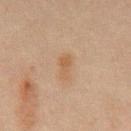Case summary:
– workup: imaged on a skin check; not biopsied
– patient: male, about 45 years old
– illumination: cross-polarized illumination
– diameter: about 3 mm
– imaging modality: total-body-photography crop, ~15 mm field of view
– site: the abdomen